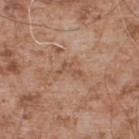The lesion's longest dimension is about 2.5 mm.
Cropped from a whole-body photographic skin survey; the tile spans about 15 mm.
The patient is a male aged 53–57.
Located on the upper back.